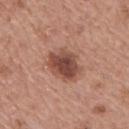Case summary:
- patient — male, aged approximately 65
- anatomic site — the upper back
- lesion size — ~4.5 mm (longest diameter)
- image source — ~15 mm crop, total-body skin-cancer survey